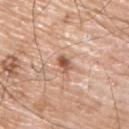Recorded during total-body skin imaging; not selected for excision or biopsy.
The tile uses white-light illumination.
Cropped from a whole-body photographic skin survey; the tile spans about 15 mm.
On the back.
A male subject, in their mid- to late 70s.